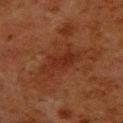<case>
<biopsy_status>not biopsied; imaged during a skin examination</biopsy_status>
<patient>
  <sex>male</sex>
  <age_approx>80</age_approx>
</patient>
<image>
  <source>total-body photography crop</source>
  <field_of_view_mm>15</field_of_view_mm>
</image>
<lesion_size>
  <long_diameter_mm_approx>5.0</long_diameter_mm_approx>
</lesion_size>
<lighting>cross-polarized</lighting>
<site>right lower leg</site>
</case>